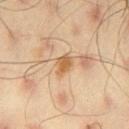Clinical impression: Imaged during a routine full-body skin examination; the lesion was not biopsied and no histopathology is available. Context: The recorded lesion diameter is about 2.5 mm. A male patient, roughly 45 years of age. The total-body-photography lesion software estimated an average lesion color of about L≈61 a*≈19 b*≈40 (CIELAB), roughly 11 lightness units darker than nearby skin, and a normalized lesion–skin contrast near 8. It also reported a nevus-likeness score of about 20/100 and lesion-presence confidence of about 100/100. Imaged with cross-polarized lighting. Located on the left thigh. A roughly 15 mm field-of-view crop from a total-body skin photograph.